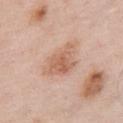Recorded during total-body skin imaging; not selected for excision or biopsy. A male subject, in their mid- to late 50s. Longest diameter approximately 4.5 mm. A region of skin cropped from a whole-body photographic capture, roughly 15 mm wide. Automated tile analysis of the lesion measured an area of roughly 11 mm², an eccentricity of roughly 0.65, and a symmetry-axis asymmetry near 0.4. And it measured a mean CIELAB color near L≈62 a*≈21 b*≈31 and about 9 CIELAB-L* units darker than the surrounding skin. It also reported a color-variation rating of about 4/10 and peripheral color asymmetry of about 1. This is a white-light tile. The lesion is on the chest.